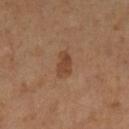Part of a total-body skin-imaging series; this lesion was reviewed on a skin check and was not flagged for biopsy.